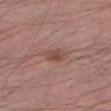Case summary:
- notes: no biopsy performed (imaged during a skin exam)
- TBP lesion metrics: an area of roughly 4.5 mm² and a shape-asymmetry score of about 0.2 (0 = symmetric); an average lesion color of about L≈48 a*≈21 b*≈26 (CIELAB), about 9 CIELAB-L* units darker than the surrounding skin, and a normalized border contrast of about 7.5; border irregularity of about 2 on a 0–10 scale and peripheral color asymmetry of about 1; a classifier nevus-likeness of about 55/100 and a detector confidence of about 100 out of 100 that the crop contains a lesion
- lesion size: about 3 mm
- subject: male, approximately 55 years of age
- acquisition: ~15 mm tile from a whole-body skin photo
- illumination: white-light
- anatomic site: the right thigh